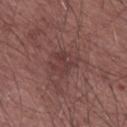Recorded during total-body skin imaging; not selected for excision or biopsy.
A male patient, in their mid-60s.
A 15 mm crop from a total-body photograph taken for skin-cancer surveillance.
On the arm.
The tile uses white-light illumination.